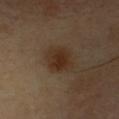{
  "biopsy_status": "not biopsied; imaged during a skin examination",
  "automated_metrics": {
    "area_mm2_approx": 8.5,
    "cielab_L": 29,
    "cielab_a": 15,
    "cielab_b": 26,
    "vs_skin_darker_L": 8.0,
    "vs_skin_contrast_norm": 9.0,
    "border_irregularity_0_10": 1.5,
    "peripheral_color_asymmetry": 0.5
  },
  "lesion_size": {
    "long_diameter_mm_approx": 3.5
  },
  "image": {
    "source": "total-body photography crop",
    "field_of_view_mm": 15
  },
  "lighting": "cross-polarized",
  "site": "chest",
  "patient": {
    "sex": "male",
    "age_approx": 40
  }
}